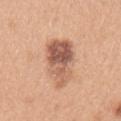Captured during whole-body skin photography for melanoma surveillance; the lesion was not biopsied. Located on the left upper arm. Cropped from a total-body skin-imaging series; the visible field is about 15 mm. A female patient approximately 30 years of age.